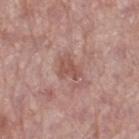Impression:
Captured during whole-body skin photography for melanoma surveillance; the lesion was not biopsied.
Clinical summary:
On the left thigh. A female patient, aged around 60. Imaged with white-light lighting. The lesion-visualizer software estimated a lesion area of about 4 mm², an eccentricity of roughly 0.7, and two-axis asymmetry of about 0.4. And it measured a lesion color around L≈52 a*≈22 b*≈25 in CIELAB, roughly 8 lightness units darker than nearby skin, and a normalized lesion–skin contrast near 6. The software also gave a border-irregularity index near 4/10, a color-variation rating of about 1.5/10, and radial color variation of about 0.5. And it measured a nevus-likeness score of about 0/100 and a detector confidence of about 100 out of 100 that the crop contains a lesion. A 15 mm crop from a total-body photograph taken for skin-cancer surveillance. The recorded lesion diameter is about 3 mm.